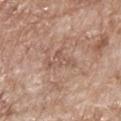Impression:
Captured during whole-body skin photography for melanoma surveillance; the lesion was not biopsied.
Context:
The recorded lesion diameter is about 4 mm. The lesion is located on the upper back. Cropped from a whole-body photographic skin survey; the tile spans about 15 mm. The total-body-photography lesion software estimated a shape eccentricity near 0.8. It also reported a mean CIELAB color near L≈55 a*≈19 b*≈27 and roughly 7 lightness units darker than nearby skin. It also reported a color-variation rating of about 1.5/10 and peripheral color asymmetry of about 0.5. A male subject, about 65 years old. This is a white-light tile.